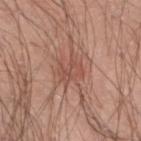Q: Is there a histopathology result?
A: imaged on a skin check; not biopsied
Q: Patient demographics?
A: male, approximately 40 years of age
Q: What kind of image is this?
A: total-body-photography crop, ~15 mm field of view
Q: Lesion location?
A: the left lower leg